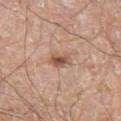Assessment: The lesion was tiled from a total-body skin photograph and was not biopsied. Clinical summary: A 15 mm close-up tile from a total-body photography series done for melanoma screening. From the right thigh. The tile uses white-light illumination. Automated image analysis of the tile measured an outline eccentricity of about 0.75 (0 = round, 1 = elongated) and two-axis asymmetry of about 0.25. And it measured a border-irregularity rating of about 2.5/10, a color-variation rating of about 6/10, and radial color variation of about 2. The analysis additionally found an automated nevus-likeness rating near 90 out of 100 and a lesion-detection confidence of about 100/100. The patient is a male aged 78 to 82. About 3 mm across.